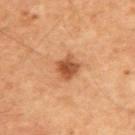The lesion was tiled from a total-body skin photograph and was not biopsied. Located on the upper back. A 15 mm close-up tile from a total-body photography series done for melanoma screening. The lesion's longest dimension is about 2.5 mm. Automated image analysis of the tile measured an area of roughly 5.5 mm², an outline eccentricity of about 0.2 (0 = round, 1 = elongated), and two-axis asymmetry of about 0.25. The analysis additionally found a within-lesion color-variation index near 3.5/10. It also reported an automated nevus-likeness rating near 95 out of 100. Captured under cross-polarized illumination. The subject is a male roughly 60 years of age.